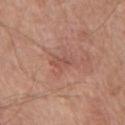follow-up: no biopsy performed (imaged during a skin exam)
illumination: white-light illumination
acquisition: ~15 mm crop, total-body skin-cancer survey
anatomic site: the front of the torso
automated lesion analysis: an area of roughly 3.5 mm², a shape eccentricity near 0.9, and two-axis asymmetry of about 0.25; a mean CIELAB color near L≈52 a*≈24 b*≈27 and a lesion-to-skin contrast of about 5 (normalized; higher = more distinct); a border-irregularity index near 3/10 and internal color variation of about 1.5 on a 0–10 scale
diameter: ≈3 mm
patient: male, aged 63 to 67